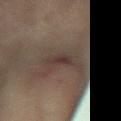Clinical impression: No biopsy was performed on this lesion — it was imaged during a full skin examination and was not determined to be concerning. Image and clinical context: The subject is a female aged around 80. The lesion-visualizer software estimated a lesion color around L≈31 a*≈13 b*≈17 in CIELAB and a normalized border contrast of about 7. The tile uses cross-polarized illumination. Cropped from a whole-body photographic skin survey; the tile spans about 15 mm. Located on the arm. The lesion's longest dimension is about 3 mm.